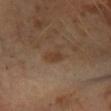Recorded during total-body skin imaging; not selected for excision or biopsy.
Automated tile analysis of the lesion measured a lesion area of about 3.5 mm² and a symmetry-axis asymmetry near 0.55.
Imaged with cross-polarized lighting.
From the left leg.
Longest diameter approximately 3 mm.
A lesion tile, about 15 mm wide, cut from a 3D total-body photograph.
The subject is a male about 30 years old.